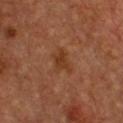A male patient, aged approximately 50. A 15 mm crop from a total-body photograph taken for skin-cancer surveillance. On the chest. This is a cross-polarized tile. Measured at roughly 3.5 mm in maximum diameter.